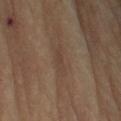Captured during whole-body skin photography for melanoma surveillance; the lesion was not biopsied. An algorithmic analysis of the crop reported a border-irregularity index near 3.5/10, a color-variation rating of about 0/10, and a peripheral color-asymmetry measure near 0. The software also gave a classifier nevus-likeness of about 0/100 and a detector confidence of about 65 out of 100 that the crop contains a lesion. The recorded lesion diameter is about 3 mm. This is a cross-polarized tile. A male patient aged 83 or over. Cropped from a total-body skin-imaging series; the visible field is about 15 mm. The lesion is on the left upper arm.